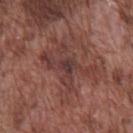| feature | finding |
|---|---|
| biopsy status | imaged on a skin check; not biopsied |
| image-analysis metrics | a footprint of about 19 mm², an eccentricity of roughly 0.7, and a shape-asymmetry score of about 0.5 (0 = symmetric); an average lesion color of about L≈39 a*≈22 b*≈22 (CIELAB), roughly 8 lightness units darker than nearby skin, and a lesion-to-skin contrast of about 6.5 (normalized; higher = more distinct); a within-lesion color-variation index near 5.5/10 |
| image source | ~15 mm tile from a whole-body skin photo |
| diameter | ≈7 mm |
| patient | male, aged around 75 |
| illumination | white-light illumination |
| anatomic site | the front of the torso |